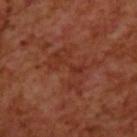The lesion was photographed on a routine skin check and not biopsied; there is no pathology result. About 5.5 mm across. A 15 mm crop from a total-body photograph taken for skin-cancer surveillance. A male patient, in their 70s. The total-body-photography lesion software estimated an area of roughly 12 mm², an eccentricity of roughly 0.8, and a symmetry-axis asymmetry near 0.45. The analysis additionally found an average lesion color of about L≈32 a*≈26 b*≈29 (CIELAB), a lesion–skin lightness drop of about 5, and a normalized lesion–skin contrast near 5. The tile uses cross-polarized illumination. The lesion is located on the back.